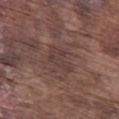The lesion was tiled from a total-body skin photograph and was not biopsied. A lesion tile, about 15 mm wide, cut from a 3D total-body photograph. Automated tile analysis of the lesion measured a lesion area of about 6 mm², an eccentricity of roughly 0.85, and two-axis asymmetry of about 0.4. The analysis additionally found roughly 5 lightness units darker than nearby skin and a normalized lesion–skin contrast near 5. The analysis additionally found an automated nevus-likeness rating near 0 out of 100 and lesion-presence confidence of about 80/100. The lesion is located on the left thigh. Measured at roughly 3.5 mm in maximum diameter. Captured under white-light illumination. A male patient in their mid-70s.